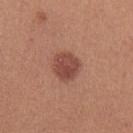Q: Was a biopsy performed?
A: catalogued during a skin exam; not biopsied
Q: What did automated image analysis measure?
A: a classifier nevus-likeness of about 95/100 and a detector confidence of about 100 out of 100 that the crop contains a lesion
Q: Who is the patient?
A: female, roughly 25 years of age
Q: What lighting was used for the tile?
A: white-light illumination
Q: What is the anatomic site?
A: the right upper arm
Q: How large is the lesion?
A: ~3.5 mm (longest diameter)
Q: What kind of image is this?
A: ~15 mm tile from a whole-body skin photo